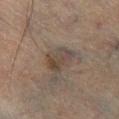The lesion was tiled from a total-body skin photograph and was not biopsied.
The lesion is on the right lower leg.
An algorithmic analysis of the crop reported a footprint of about 8 mm², an eccentricity of roughly 0.75, and a symmetry-axis asymmetry near 0.3. The software also gave a mean CIELAB color near L≈34 a*≈9 b*≈19 and a lesion–skin lightness drop of about 6. The software also gave an automated nevus-likeness rating near 0 out of 100 and a lesion-detection confidence of about 70/100.
Imaged with cross-polarized lighting.
A male patient, aged approximately 70.
Longest diameter approximately 4 mm.
A close-up tile cropped from a whole-body skin photograph, about 15 mm across.